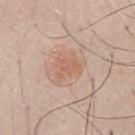workup = no biopsy performed (imaged during a skin exam) | illumination = white-light | lesion size = ~3.5 mm (longest diameter) | subject = male, approximately 50 years of age | image source = ~15 mm tile from a whole-body skin photo | location = the chest.